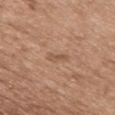Notes:
– follow-up — catalogued during a skin exam; not biopsied
– lighting — white-light
– anatomic site — the chest
– patient — male, aged 48–52
– image-analysis metrics — an area of roughly 2 mm², an eccentricity of roughly 0.95, and a symmetry-axis asymmetry near 0.45; a mean CIELAB color near L≈53 a*≈19 b*≈32, roughly 7 lightness units darker than nearby skin, and a normalized lesion–skin contrast near 5.5; a within-lesion color-variation index near 0/10; a lesion-detection confidence of about 100/100
– imaging modality — total-body-photography crop, ~15 mm field of view
– diameter — ~2.5 mm (longest diameter)